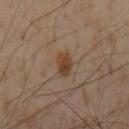About 3 mm across.
This image is a 15 mm lesion crop taken from a total-body photograph.
A male patient, aged approximately 55.
An algorithmic analysis of the crop reported a lesion area of about 4.5 mm², an eccentricity of roughly 0.8, and a shape-asymmetry score of about 0.2 (0 = symmetric).
On the mid back.